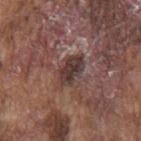{"site": "back", "lighting": "white-light", "image": {"source": "total-body photography crop", "field_of_view_mm": 15}, "patient": {"sex": "male", "age_approx": 75}, "automated_metrics": {"area_mm2_approx": 7.5, "eccentricity": 0.8, "shape_asymmetry": 0.15, "border_irregularity_0_10": 2.0, "color_variation_0_10": 4.0, "peripheral_color_asymmetry": 1.5}}